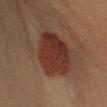biopsy_status: not biopsied; imaged during a skin examination
lesion_size:
  long_diameter_mm_approx: 7.5
lighting: cross-polarized
patient:
  sex: male
  age_approx: 40
site: left upper arm
image:
  source: total-body photography crop
  field_of_view_mm: 15